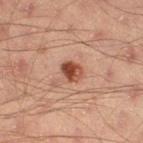This lesion was catalogued during total-body skin photography and was not selected for biopsy. The subject is a male in their mid- to late 40s. A region of skin cropped from a whole-body photographic capture, roughly 15 mm wide. Located on the right thigh.